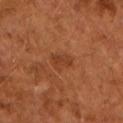  patient:
    sex: male
    age_approx: 65
  lesion_size:
    long_diameter_mm_approx: 3.0
  image:
    source: total-body photography crop
    field_of_view_mm: 15